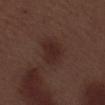| feature | finding |
|---|---|
| biopsy status | catalogued during a skin exam; not biopsied |
| TBP lesion metrics | a border-irregularity rating of about 2.5/10, a color-variation rating of about 2/10, and peripheral color asymmetry of about 0.5 |
| image | ~15 mm tile from a whole-body skin photo |
| patient | male, aged around 70 |
| lesion size | ~3.5 mm (longest diameter) |
| tile lighting | white-light |
| location | the left lower leg |